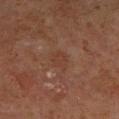{"biopsy_status": "not biopsied; imaged during a skin examination", "automated_metrics": {"nevus_likeness_0_100": 0, "lesion_detection_confidence_0_100": 100}, "image": {"source": "total-body photography crop", "field_of_view_mm": 15}, "site": "left lower leg", "lesion_size": {"long_diameter_mm_approx": 2.5}, "patient": {"sex": "male", "age_approx": 65}, "lighting": "cross-polarized"}